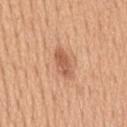Captured during whole-body skin photography for melanoma surveillance; the lesion was not biopsied. A male subject about 50 years old. Measured at roughly 4 mm in maximum diameter. A roughly 15 mm field-of-view crop from a total-body skin photograph. The lesion-visualizer software estimated a border-irregularity rating of about 2.5/10, internal color variation of about 2 on a 0–10 scale, and radial color variation of about 0.5. The lesion is on the mid back. This is a white-light tile.